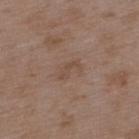follow-up: imaged on a skin check; not biopsied | body site: the back | acquisition: total-body-photography crop, ~15 mm field of view | subject: male, aged 48–52 | automated lesion analysis: a footprint of about 3 mm², a shape eccentricity near 0.9, and a symmetry-axis asymmetry near 0.45; a lesion color around L≈47 a*≈17 b*≈26 in CIELAB, a lesion–skin lightness drop of about 6, and a normalized border contrast of about 5.